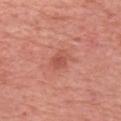No biopsy was performed on this lesion — it was imaged during a full skin examination and was not determined to be concerning. The patient is a female roughly 55 years of age. Measured at roughly 2.5 mm in maximum diameter. The tile uses white-light illumination. A lesion tile, about 15 mm wide, cut from a 3D total-body photograph. From the left forearm.